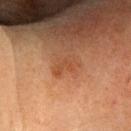follow-up — total-body-photography surveillance lesion; no biopsy | tile lighting — cross-polarized | acquisition — total-body-photography crop, ~15 mm field of view | subject — female, roughly 50 years of age | lesion diameter — about 3 mm | anatomic site — the head or neck | image-analysis metrics — a footprint of about 3 mm², an outline eccentricity of about 0.85 (0 = round, 1 = elongated), and a symmetry-axis asymmetry near 0.4; a within-lesion color-variation index near 0/10.